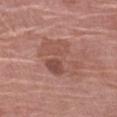No biopsy was performed on this lesion — it was imaged during a full skin examination and was not determined to be concerning.
On the left upper arm.
A female patient aged 58–62.
Longest diameter approximately 5.5 mm.
A close-up tile cropped from a whole-body skin photograph, about 15 mm across.
This is a white-light tile.